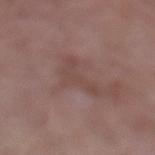workup — total-body-photography surveillance lesion; no biopsy
illumination — white-light
acquisition — ~15 mm crop, total-body skin-cancer survey
body site — the left lower leg
image-analysis metrics — an area of roughly 8 mm² and a symmetry-axis asymmetry near 0.7; border irregularity of about 8.5 on a 0–10 scale, internal color variation of about 1.5 on a 0–10 scale, and a peripheral color-asymmetry measure near 0.5
patient — female, aged around 70
lesion diameter — ≈4 mm